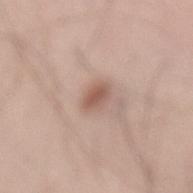workup: total-body-photography surveillance lesion; no biopsy | image: 15 mm crop, total-body photography | patient: male, aged around 60 | diameter: ~2.5 mm (longest diameter) | anatomic site: the lower back.